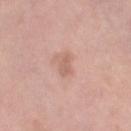The lesion was tiled from a total-body skin photograph and was not biopsied. Cropped from a whole-body photographic skin survey; the tile spans about 15 mm. Automated image analysis of the tile measured two-axis asymmetry of about 0.35. And it measured a lesion color around L≈62 a*≈21 b*≈27 in CIELAB, roughly 8 lightness units darker than nearby skin, and a normalized border contrast of about 5.5. The software also gave a lesion-detection confidence of about 100/100. The lesion is on the left thigh. Approximately 2.5 mm at its widest. A female subject in their 50s.